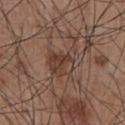{"biopsy_status": "not biopsied; imaged during a skin examination", "image": {"source": "total-body photography crop", "field_of_view_mm": 15}, "lighting": "white-light", "site": "abdomen", "patient": {"sex": "male", "age_approx": 55}}